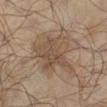{
  "biopsy_status": "not biopsied; imaged during a skin examination",
  "image": {
    "source": "total-body photography crop",
    "field_of_view_mm": 15
  },
  "lesion_size": {
    "long_diameter_mm_approx": 7.0
  },
  "patient": {
    "sex": "male",
    "age_approx": 65
  },
  "automated_metrics": {
    "cielab_L": 45,
    "cielab_a": 13,
    "cielab_b": 26,
    "vs_skin_darker_L": 8.0,
    "vs_skin_contrast_norm": 6.5
  },
  "lighting": "cross-polarized",
  "site": "left lower leg"
}